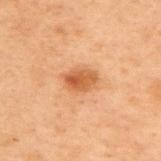Assessment: The lesion was photographed on a routine skin check and not biopsied; there is no pathology result. Acquisition and patient details: This is a cross-polarized tile. A male patient, aged around 70. This image is a 15 mm lesion crop taken from a total-body photograph. About 3.5 mm across. Automated image analysis of the tile measured an average lesion color of about L≈45 a*≈21 b*≈33 (CIELAB), roughly 9 lightness units darker than nearby skin, and a normalized lesion–skin contrast near 7.5. The software also gave a border-irregularity rating of about 2/10 and a peripheral color-asymmetry measure near 1.5. It also reported a classifier nevus-likeness of about 85/100 and a detector confidence of about 100 out of 100 that the crop contains a lesion. From the upper back.